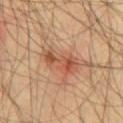Recorded during total-body skin imaging; not selected for excision or biopsy. A 15 mm crop from a total-body photograph taken for skin-cancer surveillance. Captured under cross-polarized illumination. A male subject aged around 55. From the chest.